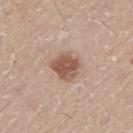Captured during whole-body skin photography for melanoma surveillance; the lesion was not biopsied.
The subject is a male aged 58 to 62.
A roughly 15 mm field-of-view crop from a total-body skin photograph.
Automated image analysis of the tile measured a lesion–skin lightness drop of about 13 and a normalized lesion–skin contrast near 9. The software also gave a nevus-likeness score of about 95/100 and a lesion-detection confidence of about 100/100.
The lesion is located on the left upper arm.
Measured at roughly 3.5 mm in maximum diameter.
Captured under white-light illumination.